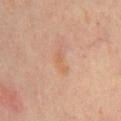{"biopsy_status": "not biopsied; imaged during a skin examination", "image": {"source": "total-body photography crop", "field_of_view_mm": 15}, "automated_metrics": {"area_mm2_approx": 2.5, "eccentricity": 0.95, "shape_asymmetry": 0.5, "border_irregularity_0_10": 5.0, "color_variation_0_10": 0.0, "peripheral_color_asymmetry": 0.0, "nevus_likeness_0_100": 0, "lesion_detection_confidence_0_100": 100}, "patient": {"sex": "female", "age_approx": 45}, "site": "chest", "lighting": "cross-polarized"}